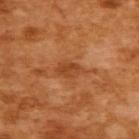Q: Was a biopsy performed?
A: imaged on a skin check; not biopsied
Q: Patient demographics?
A: female, approximately 55 years of age
Q: What did automated image analysis measure?
A: a footprint of about 5 mm² and an eccentricity of roughly 0.8; a normalized lesion–skin contrast near 6.5; a border-irregularity index near 3/10, a within-lesion color-variation index near 2.5/10, and peripheral color asymmetry of about 1
Q: What kind of image is this?
A: ~15 mm crop, total-body skin-cancer survey
Q: Lesion location?
A: the back